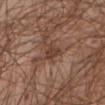Recorded during total-body skin imaging; not selected for excision or biopsy.
Cropped from a whole-body photographic skin survey; the tile spans about 15 mm.
A male patient in their mid- to late 60s.
Located on the abdomen.
The tile uses white-light illumination.
The lesion's longest dimension is about 2.5 mm.
The lesion-visualizer software estimated a footprint of about 3.5 mm², a shape eccentricity near 0.75, and a shape-asymmetry score of about 0.35 (0 = symmetric). And it measured a border-irregularity index near 3.5/10, a within-lesion color-variation index near 1/10, and radial color variation of about 0.5. The software also gave an automated nevus-likeness rating near 0 out of 100 and a lesion-detection confidence of about 95/100.